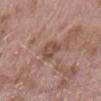This lesion was catalogued during total-body skin photography and was not selected for biopsy. A male subject, aged around 55. A 15 mm close-up extracted from a 3D total-body photography capture. The lesion's longest dimension is about 2.5 mm. This is a white-light tile. On the back.